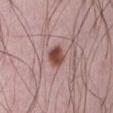• biopsy status: imaged on a skin check; not biopsied
• tile lighting: white-light illumination
• anatomic site: the abdomen
• image source: total-body-photography crop, ~15 mm field of view
• patient: male, aged approximately 70